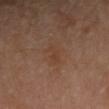| key | value |
|---|---|
| location | the left forearm |
| size | about 3 mm |
| illumination | cross-polarized illumination |
| image-analysis metrics | an area of roughly 4 mm², an eccentricity of roughly 0.9, and a symmetry-axis asymmetry near 0.3; a mean CIELAB color near L≈40 a*≈18 b*≈29, about 4 CIELAB-L* units darker than the surrounding skin, and a normalized lesion–skin contrast near 5 |
| patient | female, approximately 65 years of age |
| image | total-body-photography crop, ~15 mm field of view |